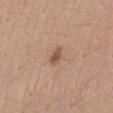The lesion is on the abdomen.
A close-up tile cropped from a whole-body skin photograph, about 15 mm across.
A male patient, roughly 30 years of age.